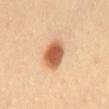Recorded during total-body skin imaging; not selected for excision or biopsy.
The lesion is located on the chest.
The patient is a male aged around 40.
Imaged with cross-polarized lighting.
A 15 mm close-up extracted from a 3D total-body photography capture.